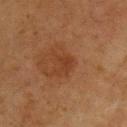Impression: Recorded during total-body skin imaging; not selected for excision or biopsy. Background: Located on the front of the torso. Longest diameter approximately 3 mm. Imaged with cross-polarized lighting. A male patient roughly 75 years of age. A 15 mm close-up extracted from a 3D total-body photography capture.